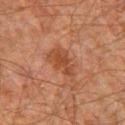Impression:
The lesion was photographed on a routine skin check and not biopsied; there is no pathology result.
Context:
This is a cross-polarized tile. A male patient in their 60s. The recorded lesion diameter is about 5 mm. Located on the chest. A 15 mm close-up extracted from a 3D total-body photography capture.